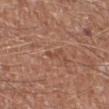No biopsy was performed on this lesion — it was imaged during a full skin examination and was not determined to be concerning.
The recorded lesion diameter is about 2.5 mm.
A male patient aged approximately 80.
A 15 mm close-up tile from a total-body photography series done for melanoma screening.
From the right lower leg.